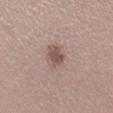* workup — catalogued during a skin exam; not biopsied
* image source — 15 mm crop, total-body photography
* anatomic site — the right lower leg
* illumination — white-light
* lesion size — ~3 mm (longest diameter)
* patient — female, approximately 45 years of age